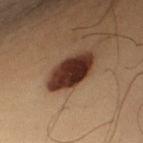{"biopsy_status": "not biopsied; imaged during a skin examination", "image": {"source": "total-body photography crop", "field_of_view_mm": 15}, "site": "left upper arm", "patient": {"sex": "male", "age_approx": 40}}